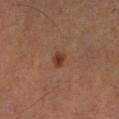Findings:
- biopsy status — total-body-photography surveillance lesion; no biopsy
- body site — the arm
- image — 15 mm crop, total-body photography
- tile lighting — cross-polarized illumination
- size — ~2 mm (longest diameter)
- patient — male, in their 60s